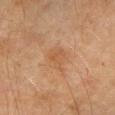Captured under cross-polarized illumination.
From the right forearm.
An algorithmic analysis of the crop reported a lesion area of about 5.5 mm², an outline eccentricity of about 0.55 (0 = round, 1 = elongated), and a symmetry-axis asymmetry near 0.3. It also reported an automated nevus-likeness rating near 0 out of 100.
The lesion's longest dimension is about 3 mm.
A lesion tile, about 15 mm wide, cut from a 3D total-body photograph.
The subject is a female about 70 years old.